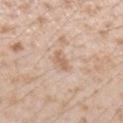The lesion was photographed on a routine skin check and not biopsied; there is no pathology result. A male patient, in their mid- to late 20s. The lesion is on the left forearm. Cropped from a whole-body photographic skin survey; the tile spans about 15 mm.